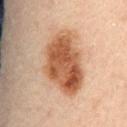image-analysis metrics: a shape-asymmetry score of about 0.2 (0 = symmetric); an average lesion color of about L≈55 a*≈23 b*≈35 (CIELAB) and a lesion–skin lightness drop of about 15; a border-irregularity rating of about 2.5/10, a within-lesion color-variation index near 7.5/10, and a peripheral color-asymmetry measure near 3; a nevus-likeness score of about 100/100 and a detector confidence of about 100 out of 100 that the crop contains a lesion
site: the mid back
illumination: cross-polarized illumination
image: 15 mm crop, total-body photography
diameter: about 8 mm
patient: male, in their mid- to late 80s or older